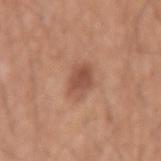subject = male, aged approximately 55
imaging modality = 15 mm crop, total-body photography
illumination = white-light
automated lesion analysis = an area of roughly 7 mm², a shape eccentricity near 0.65, and a symmetry-axis asymmetry near 0.25; a lesion color around L≈51 a*≈23 b*≈30 in CIELAB, about 10 CIELAB-L* units darker than the surrounding skin, and a normalized border contrast of about 7.5; a border-irregularity index near 2.5/10 and a color-variation rating of about 3.5/10; a classifier nevus-likeness of about 55/100
site = the left upper arm